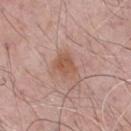Findings:
* workup — imaged on a skin check; not biopsied
* patient — male, about 65 years old
* anatomic site — the chest
* image — total-body-photography crop, ~15 mm field of view